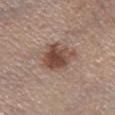Q: Is there a histopathology result?
A: imaged on a skin check; not biopsied
Q: What lighting was used for the tile?
A: white-light illumination
Q: What is the anatomic site?
A: the leg
Q: What are the patient's age and sex?
A: male, roughly 55 years of age
Q: Lesion size?
A: about 4.5 mm
Q: What is the imaging modality?
A: total-body-photography crop, ~15 mm field of view